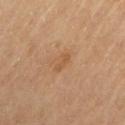workup: catalogued during a skin exam; not biopsied
image: total-body-photography crop, ~15 mm field of view
tile lighting: cross-polarized illumination
size: about 2.5 mm
patient: female, aged 63 to 67
anatomic site: the left upper arm